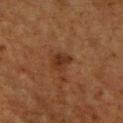Q: Is there a histopathology result?
A: imaged on a skin check; not biopsied
Q: What is the imaging modality?
A: ~15 mm tile from a whole-body skin photo
Q: Illumination type?
A: cross-polarized illumination
Q: Lesion size?
A: ~2.5 mm (longest diameter)
Q: Who is the patient?
A: male, about 60 years old
Q: Where on the body is the lesion?
A: the chest
Q: What did automated image analysis measure?
A: a mean CIELAB color near L≈27 a*≈19 b*≈26, about 7 CIELAB-L* units darker than the surrounding skin, and a lesion-to-skin contrast of about 7.5 (normalized; higher = more distinct)